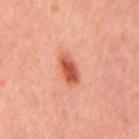Clinical impression: Recorded during total-body skin imaging; not selected for excision or biopsy. Background: A male subject aged 43 to 47. The tile uses cross-polarized illumination. Cropped from a whole-body photographic skin survey; the tile spans about 15 mm. The lesion is located on the mid back.